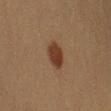| feature | finding |
|---|---|
| biopsy status | total-body-photography surveillance lesion; no biopsy |
| anatomic site | the left upper arm |
| acquisition | total-body-photography crop, ~15 mm field of view |
| lesion diameter | ~3 mm (longest diameter) |
| patient | female, aged around 40 |
| lighting | cross-polarized illumination |